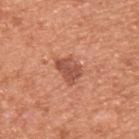The lesion was tiled from a total-body skin photograph and was not biopsied. The lesion-visualizer software estimated a border-irregularity rating of about 3.5/10 and a peripheral color-asymmetry measure near 1. The analysis additionally found an automated nevus-likeness rating near 75 out of 100 and a detector confidence of about 100 out of 100 that the crop contains a lesion. Cropped from a whole-body photographic skin survey; the tile spans about 15 mm. The subject is a male about 55 years old. From the upper back. Longest diameter approximately 3.5 mm. Captured under white-light illumination.